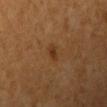This lesion was catalogued during total-body skin photography and was not selected for biopsy. This image is a 15 mm lesion crop taken from a total-body photograph. A female subject aged 63 to 67. The total-body-photography lesion software estimated an average lesion color of about L≈31 a*≈20 b*≈32 (CIELAB), roughly 7 lightness units darker than nearby skin, and a lesion-to-skin contrast of about 7.5 (normalized; higher = more distinct). It also reported internal color variation of about 0 on a 0–10 scale and radial color variation of about 0. And it measured a classifier nevus-likeness of about 75/100 and lesion-presence confidence of about 100/100. Approximately 2 mm at its widest. On the left upper arm.